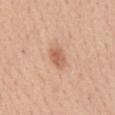Captured during whole-body skin photography for melanoma surveillance; the lesion was not biopsied. The tile uses white-light illumination. The recorded lesion diameter is about 3 mm. A female patient, aged around 55. A 15 mm close-up extracted from a 3D total-body photography capture. From the mid back.